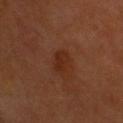{
  "biopsy_status": "not biopsied; imaged during a skin examination",
  "automated_metrics": {
    "eccentricity": 0.8,
    "shape_asymmetry": 0.25,
    "vs_skin_darker_L": 6.0,
    "vs_skin_contrast_norm": 6.5,
    "border_irregularity_0_10": 2.5
  },
  "patient": {
    "sex": "male",
    "age_approx": 60
  },
  "lesion_size": {
    "long_diameter_mm_approx": 3.0
  },
  "image": {
    "source": "total-body photography crop",
    "field_of_view_mm": 15
  },
  "site": "head or neck"
}